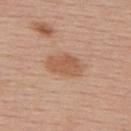Findings:
• follow-up: total-body-photography surveillance lesion; no biopsy
• TBP lesion metrics: a lesion area of about 8.5 mm² and an outline eccentricity of about 0.8 (0 = round, 1 = elongated); a within-lesion color-variation index near 2/10 and peripheral color asymmetry of about 1; a nevus-likeness score of about 85/100 and a detector confidence of about 100 out of 100 that the crop contains a lesion
• size: ≈4.5 mm
• lighting: white-light illumination
• patient: female, aged 53 to 57
• imaging modality: 15 mm crop, total-body photography
• site: the back